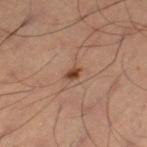Recorded during total-body skin imaging; not selected for excision or biopsy. On the leg. Cropped from a total-body skin-imaging series; the visible field is about 15 mm. The lesion-visualizer software estimated a footprint of about 2.5 mm² and a symmetry-axis asymmetry near 0.3. The analysis additionally found a border-irregularity index near 2.5/10, a color-variation rating of about 1.5/10, and a peripheral color-asymmetry measure near 0. And it measured a nevus-likeness score of about 90/100 and a lesion-detection confidence of about 100/100. This is a cross-polarized tile. A male subject, roughly 50 years of age. The lesion's longest dimension is about 2 mm.